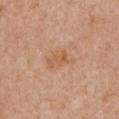No biopsy was performed on this lesion — it was imaged during a full skin examination and was not determined to be concerning.
A 15 mm close-up extracted from a 3D total-body photography capture.
A female subject, aged around 70.
The lesion's longest dimension is about 3.5 mm.
Captured under white-light illumination.
From the chest.